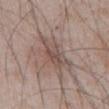Imaged during a routine full-body skin examination; the lesion was not biopsied and no histopathology is available. A male subject, aged 48 to 52. The lesion is on the abdomen. About 6 mm across. A lesion tile, about 15 mm wide, cut from a 3D total-body photograph.